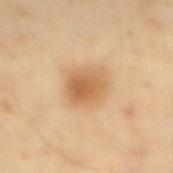The lesion was photographed on a routine skin check and not biopsied; there is no pathology result. A 15 mm close-up extracted from a 3D total-body photography capture. From the mid back. A male patient aged around 40. Captured under cross-polarized illumination. An algorithmic analysis of the crop reported a footprint of about 12 mm², an outline eccentricity of about 0.6 (0 = round, 1 = elongated), and a shape-asymmetry score of about 0.15 (0 = symmetric). The software also gave a mean CIELAB color near L≈62 a*≈20 b*≈39, a lesion–skin lightness drop of about 11, and a normalized lesion–skin contrast near 7.5. It also reported an automated nevus-likeness rating near 90 out of 100. The recorded lesion diameter is about 4 mm.